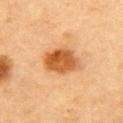Impression: This lesion was catalogued during total-body skin photography and was not selected for biopsy. Context: From the upper back. The tile uses cross-polarized illumination. A female patient aged approximately 60. A 15 mm crop from a total-body photograph taken for skin-cancer surveillance. The lesion's longest dimension is about 4.5 mm.